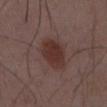This lesion was catalogued during total-body skin photography and was not selected for biopsy.
A male patient about 50 years old.
Located on the mid back.
A 15 mm close-up extracted from a 3D total-body photography capture.